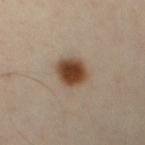Part of a total-body skin-imaging series; this lesion was reviewed on a skin check and was not flagged for biopsy. Imaged with cross-polarized lighting. The total-body-photography lesion software estimated an average lesion color of about L≈45 a*≈17 b*≈30 (CIELAB), about 16 CIELAB-L* units darker than the surrounding skin, and a normalized border contrast of about 12.5. The software also gave a border-irregularity index near 2/10 and internal color variation of about 6 on a 0–10 scale. A close-up tile cropped from a whole-body skin photograph, about 15 mm across. About 4 mm across. The lesion is located on the chest. The subject is a male roughly 40 years of age.